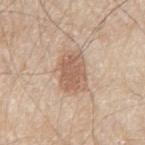This lesion was catalogued during total-body skin photography and was not selected for biopsy.
On the mid back.
Cropped from a whole-body photographic skin survey; the tile spans about 15 mm.
Captured under white-light illumination.
Measured at roughly 4.5 mm in maximum diameter.
Automated image analysis of the tile measured a lesion area of about 12 mm² and two-axis asymmetry of about 0.15. And it measured border irregularity of about 2 on a 0–10 scale, internal color variation of about 3 on a 0–10 scale, and peripheral color asymmetry of about 1.
The patient is a male about 80 years old.